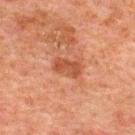This lesion was catalogued during total-body skin photography and was not selected for biopsy. Automated tile analysis of the lesion measured a border-irregularity rating of about 2/10, a within-lesion color-variation index near 2/10, and peripheral color asymmetry of about 0.5. It also reported an automated nevus-likeness rating near 10 out of 100 and a lesion-detection confidence of about 100/100. Cropped from a whole-body photographic skin survey; the tile spans about 15 mm. On the upper back. A male subject, aged 58–62.